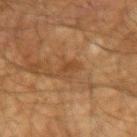Acquisition and patient details: The tile uses cross-polarized illumination. Measured at roughly 2.5 mm in maximum diameter. Automated tile analysis of the lesion measured a lesion area of about 4 mm², a shape eccentricity near 0.6, and two-axis asymmetry of about 0.25. It also reported an average lesion color of about L≈36 a*≈17 b*≈30 (CIELAB), a lesion–skin lightness drop of about 6, and a lesion-to-skin contrast of about 5.5 (normalized; higher = more distinct). The analysis additionally found lesion-presence confidence of about 95/100. A region of skin cropped from a whole-body photographic capture, roughly 15 mm wide. From the right forearm. A male subject, roughly 50 years of age.